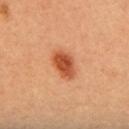{"biopsy_status": "not biopsied; imaged during a skin examination", "automated_metrics": {"cielab_L": 53, "cielab_a": 31, "cielab_b": 40, "vs_skin_contrast_norm": 9.5, "border_irregularity_0_10": 1.5, "peripheral_color_asymmetry": 1.5}, "lesion_size": {"long_diameter_mm_approx": 4.0}, "patient": {"sex": "female", "age_approx": 50}, "image": {"source": "total-body photography crop", "field_of_view_mm": 15}}